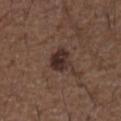Recorded during total-body skin imaging; not selected for excision or biopsy. The tile uses white-light illumination. On the right upper arm. Cropped from a whole-body photographic skin survey; the tile spans about 15 mm. Automated image analysis of the tile measured an average lesion color of about L≈30 a*≈16 b*≈19 (CIELAB) and roughly 10 lightness units darker than nearby skin. The analysis additionally found border irregularity of about 1.5 on a 0–10 scale, a within-lesion color-variation index near 4.5/10, and peripheral color asymmetry of about 1.5. And it measured a classifier nevus-likeness of about 40/100 and lesion-presence confidence of about 100/100. A male patient, aged approximately 50. The lesion's longest dimension is about 3 mm.